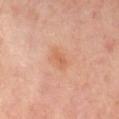Notes:
– notes · catalogued during a skin exam; not biopsied
– tile lighting · cross-polarized illumination
– TBP lesion metrics · a lesion area of about 3 mm², a shape eccentricity near 0.8, and two-axis asymmetry of about 0.3; a mean CIELAB color near L≈59 a*≈24 b*≈34, a lesion–skin lightness drop of about 7, and a lesion-to-skin contrast of about 5.5 (normalized; higher = more distinct); border irregularity of about 2.5 on a 0–10 scale, internal color variation of about 1 on a 0–10 scale, and a peripheral color-asymmetry measure near 0.5; a classifier nevus-likeness of about 10/100 and a detector confidence of about 100 out of 100 that the crop contains a lesion
– acquisition · 15 mm crop, total-body photography
– subject · male, aged 63–67
– anatomic site · the mid back
– diameter · about 2.5 mm